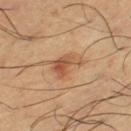No biopsy was performed on this lesion — it was imaged during a full skin examination and was not determined to be concerning.
A close-up tile cropped from a whole-body skin photograph, about 15 mm across.
The lesion's longest dimension is about 4 mm.
A male subject, about 65 years old.
Located on the left thigh.
This is a cross-polarized tile.
The total-body-photography lesion software estimated a mean CIELAB color near L≈55 a*≈22 b*≈35, roughly 10 lightness units darker than nearby skin, and a normalized lesion–skin contrast near 7. The software also gave border irregularity of about 4 on a 0–10 scale, internal color variation of about 6 on a 0–10 scale, and peripheral color asymmetry of about 2.